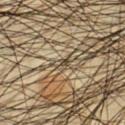This lesion was catalogued during total-body skin photography and was not selected for biopsy.
A lesion tile, about 15 mm wide, cut from a 3D total-body photograph.
A male subject approximately 45 years of age.
On the front of the torso.